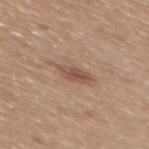The lesion was photographed on a routine skin check and not biopsied; there is no pathology result. A female subject, aged 43–47. This is a white-light tile. Cropped from a total-body skin-imaging series; the visible field is about 15 mm. Measured at roughly 3.5 mm in maximum diameter. The lesion is located on the mid back.